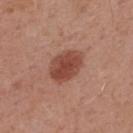<tbp_lesion>
<biopsy_status>not biopsied; imaged during a skin examination</biopsy_status>
<patient>
  <sex>male</sex>
  <age_approx>55</age_approx>
</patient>
<lighting>white-light</lighting>
<image>
  <source>total-body photography crop</source>
  <field_of_view_mm>15</field_of_view_mm>
</image>
<site>upper back</site>
<lesion_size>
  <long_diameter_mm_approx>4.5</long_diameter_mm_approx>
</lesion_size>
<automated_metrics>
  <peripheral_color_asymmetry>1.0</peripheral_color_asymmetry>
</automated_metrics>
</tbp_lesion>